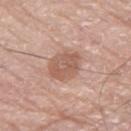Q: Is there a histopathology result?
A: no biopsy performed (imaged during a skin exam)
Q: Lesion size?
A: ≈4 mm
Q: What did automated image analysis measure?
A: a footprint of about 11 mm², an outline eccentricity of about 0.6 (0 = round, 1 = elongated), and a shape-asymmetry score of about 0.15 (0 = symmetric); a lesion–skin lightness drop of about 10 and a lesion-to-skin contrast of about 7 (normalized; higher = more distinct); border irregularity of about 1.5 on a 0–10 scale, a within-lesion color-variation index near 3.5/10, and radial color variation of about 1
Q: How was this image acquired?
A: ~15 mm tile from a whole-body skin photo
Q: What are the patient's age and sex?
A: male, in their 80s
Q: Lesion location?
A: the right thigh
Q: Illumination type?
A: white-light